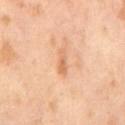Captured during whole-body skin photography for melanoma surveillance; the lesion was not biopsied.
A male patient, roughly 65 years of age.
A 15 mm close-up extracted from a 3D total-body photography capture.